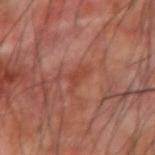Assessment: This lesion was catalogued during total-body skin photography and was not selected for biopsy. Image and clinical context: A roughly 15 mm field-of-view crop from a total-body skin photograph. A male subject, in their 70s. The total-body-photography lesion software estimated a lesion color around L≈43 a*≈27 b*≈31 in CIELAB, about 6 CIELAB-L* units darker than the surrounding skin, and a lesion-to-skin contrast of about 5.5 (normalized; higher = more distinct). It also reported an automated nevus-likeness rating near 0 out of 100. From the left forearm. Imaged with cross-polarized lighting. Longest diameter approximately 3 mm.